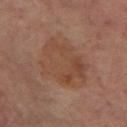biopsy status: no biopsy performed (imaged during a skin exam) | diameter: ~6.5 mm (longest diameter) | image source: total-body-photography crop, ~15 mm field of view | body site: the right leg | patient: female, aged 63–67 | tile lighting: cross-polarized illumination | automated metrics: a lesion area of about 28 mm², an outline eccentricity of about 0.55 (0 = round, 1 = elongated), and a shape-asymmetry score of about 0.2 (0 = symmetric); a mean CIELAB color near L≈44 a*≈19 b*≈28, roughly 5 lightness units darker than nearby skin, and a lesion-to-skin contrast of about 5.5 (normalized; higher = more distinct); a nevus-likeness score of about 0/100 and a detector confidence of about 100 out of 100 that the crop contains a lesion.